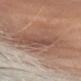imaging modality: total-body-photography crop, ~15 mm field of view
automated lesion analysis: an area of roughly 33 mm², a shape eccentricity near 0.85, and a shape-asymmetry score of about 0.35 (0 = symmetric); a nevus-likeness score of about 0/100 and lesion-presence confidence of about 70/100
lesion diameter: ≈9.5 mm
patient: female, roughly 75 years of age
body site: the head or neck
tile lighting: cross-polarized